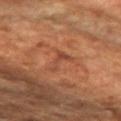The lesion was photographed on a routine skin check and not biopsied; there is no pathology result.
The recorded lesion diameter is about 3.5 mm.
A female subject in their 70s.
Captured under cross-polarized illumination.
The total-body-photography lesion software estimated a classifier nevus-likeness of about 0/100.
This image is a 15 mm lesion crop taken from a total-body photograph.
From the right forearm.